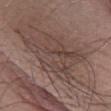The lesion was tiled from a total-body skin photograph and was not biopsied. The subject is a male aged 73–77. Cropped from a whole-body photographic skin survey; the tile spans about 15 mm. On the left lower leg. The tile uses white-light illumination.